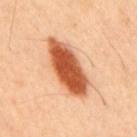| key | value |
|---|---|
| patient | male, approximately 45 years of age |
| location | the upper back |
| lesion size | ≈8 mm |
| TBP lesion metrics | a mean CIELAB color near L≈54 a*≈29 b*≈38, roughly 19 lightness units darker than nearby skin, and a normalized border contrast of about 12.5; a border-irregularity rating of about 2.5/10 and a peripheral color-asymmetry measure near 1.5; a classifier nevus-likeness of about 100/100 and lesion-presence confidence of about 100/100 |
| imaging modality | 15 mm crop, total-body photography |
| tile lighting | cross-polarized illumination |